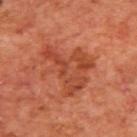biopsy_status: not biopsied; imaged during a skin examination
lesion_size:
  long_diameter_mm_approx: 6.5
automated_metrics:
  border_irregularity_0_10: 7.0
  color_variation_0_10: 5.0
  peripheral_color_asymmetry: 1.5
  nevus_likeness_0_100: 15
  lesion_detection_confidence_0_100: 100
site: upper back
image:
  source: total-body photography crop
  field_of_view_mm: 15
patient:
  sex: male
  age_approx: 70
lighting: cross-polarized